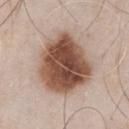– workup: total-body-photography surveillance lesion; no biopsy
– body site: the chest
– size: about 7 mm
– patient: male, in their mid-50s
– acquisition: total-body-photography crop, ~15 mm field of view